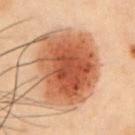Impression: No biopsy was performed on this lesion — it was imaged during a full skin examination and was not determined to be concerning. Clinical summary: A male subject roughly 55 years of age. An algorithmic analysis of the crop reported a footprint of about 44 mm², an eccentricity of roughly 0.35, and a shape-asymmetry score of about 0.15 (0 = symmetric). And it measured a mean CIELAB color near L≈45 a*≈23 b*≈31. The analysis additionally found a border-irregularity index near 2/10, a color-variation rating of about 6/10, and radial color variation of about 1.5. The lesion is on the front of the torso. The tile uses cross-polarized illumination. The lesion's longest dimension is about 8 mm. Cropped from a total-body skin-imaging series; the visible field is about 15 mm.